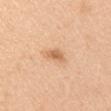Q: Is there a histopathology result?
A: imaged on a skin check; not biopsied